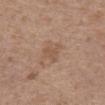The lesion was tiled from a total-body skin photograph and was not biopsied.